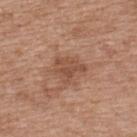The lesion's longest dimension is about 3.5 mm. This is a white-light tile. Cropped from a whole-body photographic skin survey; the tile spans about 15 mm. A female patient about 40 years old. The lesion is located on the upper back.